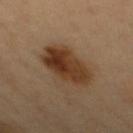Findings:
- image · total-body-photography crop, ~15 mm field of view
- illumination · cross-polarized
- subject · male, in their 50s
- site · the chest
- automated metrics · about 12 CIELAB-L* units darker than the surrounding skin and a normalized lesion–skin contrast near 11; a within-lesion color-variation index near 6.5/10; a nevus-likeness score of about 100/100